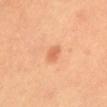Q: Was a biopsy performed?
A: catalogued during a skin exam; not biopsied
Q: Illumination type?
A: cross-polarized illumination
Q: What is the imaging modality?
A: 15 mm crop, total-body photography
Q: Lesion size?
A: about 2 mm
Q: What is the anatomic site?
A: the back
Q: Patient demographics?
A: female, aged around 30